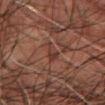follow-up = catalogued during a skin exam; not biopsied
lesion size = about 3 mm
lighting = cross-polarized illumination
acquisition = total-body-photography crop, ~15 mm field of view
body site = the abdomen
patient = male, aged 83 or over